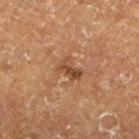The lesion was photographed on a routine skin check and not biopsied; there is no pathology result.
This is a cross-polarized tile.
The lesion is located on the leg.
The subject is a male approximately 75 years of age.
Approximately 3 mm at its widest.
A 15 mm close-up extracted from a 3D total-body photography capture.